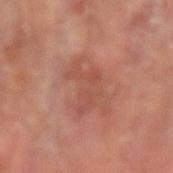biopsy_status: not biopsied; imaged during a skin examination
site: right forearm
image:
  source: total-body photography crop
  field_of_view_mm: 15
patient:
  sex: male
  age_approx: 65